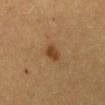Notes:
– biopsy status: total-body-photography surveillance lesion; no biopsy
– patient: female, roughly 55 years of age
– body site: the abdomen
– imaging modality: total-body-photography crop, ~15 mm field of view